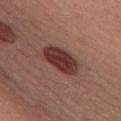Q: Was this lesion biopsied?
A: catalogued during a skin exam; not biopsied
Q: Where on the body is the lesion?
A: the chest
Q: What is the imaging modality?
A: 15 mm crop, total-body photography
Q: Who is the patient?
A: female, about 40 years old